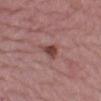The lesion was photographed on a routine skin check and not biopsied; there is no pathology result. A 15 mm close-up extracted from a 3D total-body photography capture. About 3 mm across. A female patient roughly 65 years of age. On the left lower leg.